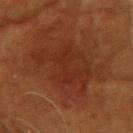Assessment:
Recorded during total-body skin imaging; not selected for excision or biopsy.
Background:
A female patient, roughly 80 years of age. This is a cross-polarized tile. From the head or neck. Longest diameter approximately 7.5 mm. Cropped from a whole-body photographic skin survey; the tile spans about 15 mm. Automated tile analysis of the lesion measured an average lesion color of about L≈22 a*≈19 b*≈24 (CIELAB) and about 4 CIELAB-L* units darker than the surrounding skin.